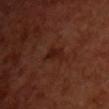Assessment:
Imaged during a routine full-body skin examination; the lesion was not biopsied and no histopathology is available.
Clinical summary:
Captured under cross-polarized illumination. A 15 mm close-up extracted from a 3D total-body photography capture. A female subject, aged around 55. On the chest. Longest diameter approximately 2.5 mm.